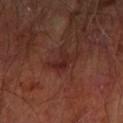– notes · no biopsy performed (imaged during a skin exam)